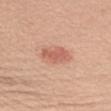Clinical impression: The lesion was photographed on a routine skin check and not biopsied; there is no pathology result. Background: A close-up tile cropped from a whole-body skin photograph, about 15 mm across. About 3.5 mm across. Located on the left upper arm. This is a white-light tile. A female subject, approximately 45 years of age.